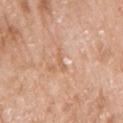biopsy status: catalogued during a skin exam; not biopsied | subject: female, aged around 75 | site: the right upper arm | acquisition: 15 mm crop, total-body photography.